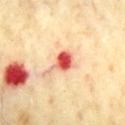site: chest
image:
  source: total-body photography crop
  field_of_view_mm: 15
lighting: cross-polarized
patient:
  sex: male
  age_approx: 65